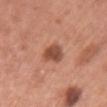Captured during whole-body skin photography for melanoma surveillance; the lesion was not biopsied.
Located on the chest.
Automated tile analysis of the lesion measured a lesion-to-skin contrast of about 9.5 (normalized; higher = more distinct). The software also gave border irregularity of about 2.5 on a 0–10 scale, a color-variation rating of about 3.5/10, and peripheral color asymmetry of about 1.5.
Captured under white-light illumination.
This image is a 15 mm lesion crop taken from a total-body photograph.
A female patient, aged 58–62.